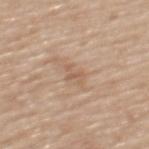A 15 mm close-up tile from a total-body photography series done for melanoma screening.
From the upper back.
A female patient about 70 years old.